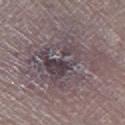notes: catalogued during a skin exam; not biopsied | location: the right lower leg | acquisition: ~15 mm tile from a whole-body skin photo | diameter: ~9 mm (longest diameter) | illumination: white-light | subject: male, in their mid- to late 70s.